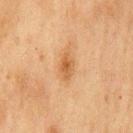The lesion is on the chest.
Captured under cross-polarized illumination.
This image is a 15 mm lesion crop taken from a total-body photograph.
A male subject, aged 73 to 77.
Automated tile analysis of the lesion measured roughly 8 lightness units darker than nearby skin and a normalized lesion–skin contrast near 7. It also reported a border-irregularity rating of about 3/10, internal color variation of about 3 on a 0–10 scale, and peripheral color asymmetry of about 1.
About 3 mm across.